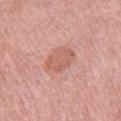The lesion was tiled from a total-body skin photograph and was not biopsied. The total-body-photography lesion software estimated about 7 CIELAB-L* units darker than the surrounding skin. It also reported a border-irregularity rating of about 1.5/10, internal color variation of about 2 on a 0–10 scale, and peripheral color asymmetry of about 0.5. The lesion is on the right thigh. A female subject, aged around 70. A 15 mm crop from a total-body photograph taken for skin-cancer surveillance.